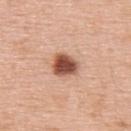- workup — no biopsy performed (imaged during a skin exam)
- acquisition — 15 mm crop, total-body photography
- size — ≈3.5 mm
- location — the upper back
- subject — male, aged approximately 60